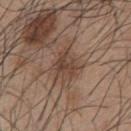The tile uses white-light illumination. The lesion-visualizer software estimated a mean CIELAB color near L≈44 a*≈17 b*≈26. The analysis additionally found a border-irregularity rating of about 3.5/10, internal color variation of about 4 on a 0–10 scale, and peripheral color asymmetry of about 1.5. The software also gave a nevus-likeness score of about 5/100 and a lesion-detection confidence of about 90/100. The lesion is on the chest. A male patient approximately 45 years of age. A lesion tile, about 15 mm wide, cut from a 3D total-body photograph.